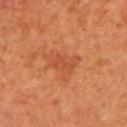workup: no biopsy performed (imaged during a skin exam); diameter: about 3.5 mm; image: ~15 mm tile from a whole-body skin photo; tile lighting: cross-polarized; site: the left upper arm; patient: female, aged 63 to 67.